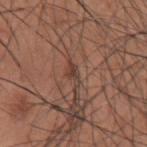Findings:
– notes: total-body-photography surveillance lesion; no biopsy
– imaging modality: ~15 mm tile from a whole-body skin photo
– diameter: ~3 mm (longest diameter)
– patient: male, in their mid- to late 30s
– site: the left upper arm
– tile lighting: white-light